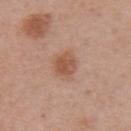biopsy status = total-body-photography surveillance lesion; no biopsy
patient = male, aged 68–72
lesion size = about 3 mm
acquisition = ~15 mm tile from a whole-body skin photo
tile lighting = white-light
body site = the abdomen
image-analysis metrics = a mean CIELAB color near L≈53 a*≈22 b*≈31, about 9 CIELAB-L* units darker than the surrounding skin, and a normalized border contrast of about 7; a classifier nevus-likeness of about 80/100 and a lesion-detection confidence of about 100/100